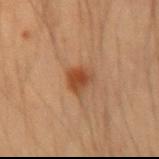Imaged during a routine full-body skin examination; the lesion was not biopsied and no histopathology is available. Measured at roughly 3 mm in maximum diameter. Cropped from a total-body skin-imaging series; the visible field is about 15 mm. The lesion is on the right forearm. A male patient, aged 33 to 37.